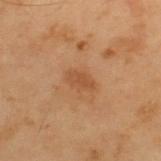Impression:
Part of a total-body skin-imaging series; this lesion was reviewed on a skin check and was not flagged for biopsy.
Clinical summary:
A 15 mm crop from a total-body photograph taken for skin-cancer surveillance. Captured under cross-polarized illumination. Located on the back. A male subject approximately 55 years of age. Automated tile analysis of the lesion measured an eccentricity of roughly 0.85 and a shape-asymmetry score of about 0.2 (0 = symmetric). It also reported a mean CIELAB color near L≈41 a*≈19 b*≈31, roughly 6 lightness units darker than nearby skin, and a normalized lesion–skin contrast near 5.5. It also reported a lesion-detection confidence of about 100/100.